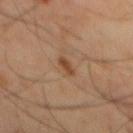Recorded during total-body skin imaging; not selected for excision or biopsy. The tile uses cross-polarized illumination. The lesion is located on the mid back. A roughly 15 mm field-of-view crop from a total-body skin photograph. An algorithmic analysis of the crop reported border irregularity of about 3 on a 0–10 scale and a within-lesion color-variation index near 1/10. The software also gave an automated nevus-likeness rating near 55 out of 100 and a detector confidence of about 100 out of 100 that the crop contains a lesion. A male subject, about 45 years old.